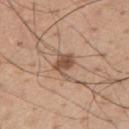Part of a total-body skin-imaging series; this lesion was reviewed on a skin check and was not flagged for biopsy. Located on the arm. The recorded lesion diameter is about 3.5 mm. A roughly 15 mm field-of-view crop from a total-body skin photograph. This is a white-light tile. The subject is a male in their mid-50s.